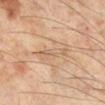follow-up=no biopsy performed (imaged during a skin exam) | subject=male, aged approximately 45 | illumination=cross-polarized | anatomic site=the leg | image=total-body-photography crop, ~15 mm field of view.